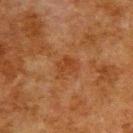• notes — imaged on a skin check; not biopsied
• TBP lesion metrics — an outline eccentricity of about 0.7 (0 = round, 1 = elongated); a border-irregularity rating of about 3.5/10 and internal color variation of about 2 on a 0–10 scale
• size — ≈3 mm
• patient — male, aged around 80
• location — the upper back
• image source — ~15 mm tile from a whole-body skin photo
• tile lighting — cross-polarized illumination